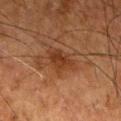Context:
Imaged with cross-polarized lighting. A roughly 15 mm field-of-view crop from a total-body skin photograph. About 3.5 mm across. The lesion is located on the right thigh. A male subject about 80 years old.